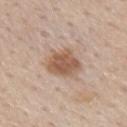biopsy status: no biopsy performed (imaged during a skin exam) | imaging modality: 15 mm crop, total-body photography | site: the mid back | patient: male, roughly 60 years of age.